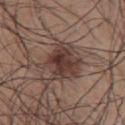Impression:
Imaged during a routine full-body skin examination; the lesion was not biopsied and no histopathology is available.
Clinical summary:
This is a white-light tile. From the upper back. A male subject about 50 years old. A close-up tile cropped from a whole-body skin photograph, about 15 mm across. Automated image analysis of the tile measured a footprint of about 15 mm² and a shape-asymmetry score of about 0.25 (0 = symmetric). It also reported a lesion color around L≈38 a*≈17 b*≈21 in CIELAB, roughly 11 lightness units darker than nearby skin, and a normalized border contrast of about 9.5. The analysis additionally found lesion-presence confidence of about 100/100.